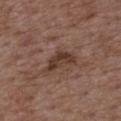This lesion was catalogued during total-body skin photography and was not selected for biopsy.
On the back.
Measured at roughly 4.5 mm in maximum diameter.
An algorithmic analysis of the crop reported a lesion color around L≈37 a*≈18 b*≈25 in CIELAB, a lesion–skin lightness drop of about 10, and a lesion-to-skin contrast of about 9 (normalized; higher = more distinct). The analysis additionally found a color-variation rating of about 3/10 and radial color variation of about 1.
A region of skin cropped from a whole-body photographic capture, roughly 15 mm wide.
A male subject, aged approximately 50.